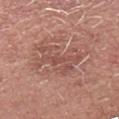Q: Is there a histopathology result?
A: imaged on a skin check; not biopsied
Q: What kind of image is this?
A: ~15 mm tile from a whole-body skin photo
Q: What is the anatomic site?
A: the head or neck
Q: Who is the patient?
A: male, aged 58 to 62